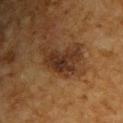Findings:
* notes: catalogued during a skin exam; not biopsied
* patient: male, roughly 60 years of age
* size: ~4 mm (longest diameter)
* lighting: cross-polarized illumination
* location: the upper back
* image: total-body-photography crop, ~15 mm field of view